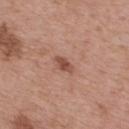Notes:
* workup: catalogued during a skin exam; not biopsied
* subject: male, aged 63 to 67
* site: the upper back
* illumination: white-light
* size: ~2.5 mm (longest diameter)
* automated lesion analysis: a footprint of about 3 mm², a shape eccentricity near 0.8, and a symmetry-axis asymmetry near 0.2; a mean CIELAB color near L≈50 a*≈23 b*≈28, a lesion–skin lightness drop of about 11, and a normalized border contrast of about 8; border irregularity of about 2 on a 0–10 scale, internal color variation of about 2.5 on a 0–10 scale, and a peripheral color-asymmetry measure near 0.5; an automated nevus-likeness rating near 55 out of 100 and a detector confidence of about 100 out of 100 that the crop contains a lesion
* image source: 15 mm crop, total-body photography